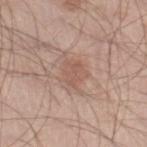biopsy status: no biopsy performed (imaged during a skin exam) | illumination: white-light | image-analysis metrics: an average lesion color of about L≈56 a*≈20 b*≈26 (CIELAB), about 7 CIELAB-L* units darker than the surrounding skin, and a lesion-to-skin contrast of about 5 (normalized; higher = more distinct) | patient: male, approximately 35 years of age | image: 15 mm crop, total-body photography | anatomic site: the right lower leg.